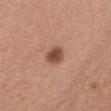{
  "biopsy_status": "not biopsied; imaged during a skin examination",
  "image": {
    "source": "total-body photography crop",
    "field_of_view_mm": 15
  },
  "lesion_size": {
    "long_diameter_mm_approx": 2.5
  },
  "lighting": "white-light",
  "automated_metrics": {
    "area_mm2_approx": 5.0,
    "eccentricity": 0.5,
    "shape_asymmetry": 0.25,
    "cielab_L": 49,
    "cielab_a": 23,
    "cielab_b": 29,
    "vs_skin_darker_L": 13.0,
    "border_irregularity_0_10": 2.0,
    "color_variation_0_10": 3.5
  },
  "site": "chest",
  "patient": {
    "sex": "female",
    "age_approx": 40
  }
}